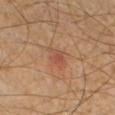Recorded during total-body skin imaging; not selected for excision or biopsy.